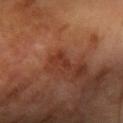follow-up: imaged on a skin check; not biopsied | location: the right forearm | subject: female, in their 70s | TBP lesion metrics: a lesion color around L≈33 a*≈26 b*≈29 in CIELAB, a lesion–skin lightness drop of about 7, and a lesion-to-skin contrast of about 6.5 (normalized; higher = more distinct); border irregularity of about 4.5 on a 0–10 scale, a color-variation rating of about 0/10, and a peripheral color-asymmetry measure near 0; a nevus-likeness score of about 0/100 | illumination: cross-polarized | acquisition: ~15 mm crop, total-body skin-cancer survey.